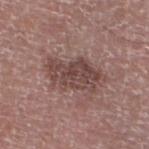Clinical impression: The lesion was photographed on a routine skin check and not biopsied; there is no pathology result. Context: A region of skin cropped from a whole-body photographic capture, roughly 15 mm wide. Located on the left lower leg. The tile uses white-light illumination. The lesion's longest dimension is about 6 mm. The patient is a male roughly 75 years of age. Automated image analysis of the tile measured a lesion-detection confidence of about 100/100.